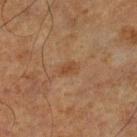Recorded during total-body skin imaging; not selected for excision or biopsy.
The lesion's longest dimension is about 2.5 mm.
A roughly 15 mm field-of-view crop from a total-body skin photograph.
Imaged with cross-polarized lighting.
The lesion is located on the right lower leg.
A male patient, approximately 65 years of age.
Automated image analysis of the tile measured lesion-presence confidence of about 100/100.